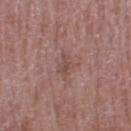Case summary:
– follow-up: total-body-photography surveillance lesion; no biopsy
– lesion size: ≈2.5 mm
– anatomic site: the right thigh
– subject: female, in their 50s
– tile lighting: white-light illumination
– imaging modality: ~15 mm crop, total-body skin-cancer survey
– image-analysis metrics: a footprint of about 3.5 mm², a shape eccentricity near 0.8, and a shape-asymmetry score of about 0.4 (0 = symmetric); a border-irregularity index near 4/10, a within-lesion color-variation index near 1/10, and peripheral color asymmetry of about 0; lesion-presence confidence of about 100/100